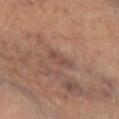The lesion was photographed on a routine skin check and not biopsied; there is no pathology result.
The subject is a female in their mid- to late 60s.
Captured under cross-polarized illumination.
The lesion's longest dimension is about 3 mm.
A region of skin cropped from a whole-body photographic capture, roughly 15 mm wide.
Located on the left lower leg.